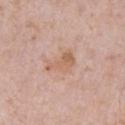{"lighting": "white-light", "lesion_size": {"long_diameter_mm_approx": 4.0}, "patient": {"sex": "male", "age_approx": 70}, "site": "left upper arm", "automated_metrics": {"area_mm2_approx": 6.5, "eccentricity": 0.85, "shape_asymmetry": 0.5}, "image": {"source": "total-body photography crop", "field_of_view_mm": 15}}